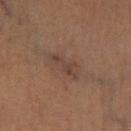<lesion>
  <biopsy_status>not biopsied; imaged during a skin examination</biopsy_status>
  <lighting>cross-polarized</lighting>
  <lesion_size>
    <long_diameter_mm_approx>4.0</long_diameter_mm_approx>
  </lesion_size>
  <patient>
    <sex>female</sex>
    <age_approx>65</age_approx>
  </patient>
  <site>left lower leg</site>
  <automated_metrics>
    <area_mm2_approx>4.5</area_mm2_approx>
    <eccentricity>0.95</eccentricity>
    <shape_asymmetry>0.4</shape_asymmetry>
    <border_irregularity_0_10>6.0</border_irregularity_0_10>
    <color_variation_0_10>1.0</color_variation_0_10>
    <peripheral_color_asymmetry>0.5</peripheral_color_asymmetry>
  </automated_metrics>
  <image>
    <source>total-body photography crop</source>
    <field_of_view_mm>15</field_of_view_mm>
  </image>
</lesion>